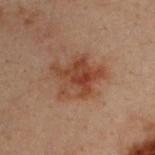Q: Is there a histopathology result?
A: imaged on a skin check; not biopsied
Q: How large is the lesion?
A: ~5.5 mm (longest diameter)
Q: What are the patient's age and sex?
A: male, roughly 30 years of age
Q: Automated lesion metrics?
A: an average lesion color of about L≈35 a*≈19 b*≈26 (CIELAB), a lesion–skin lightness drop of about 8, and a lesion-to-skin contrast of about 8 (normalized; higher = more distinct); a border-irregularity rating of about 5/10, a within-lesion color-variation index near 5.5/10, and peripheral color asymmetry of about 2
Q: What is the anatomic site?
A: the upper back
Q: What lighting was used for the tile?
A: cross-polarized illumination
Q: What is the imaging modality?
A: 15 mm crop, total-body photography